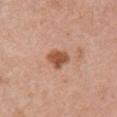workup — imaged on a skin check; not biopsied
patient — female, in their 50s
body site — the chest
image source — total-body-photography crop, ~15 mm field of view
automated lesion analysis — a classifier nevus-likeness of about 90/100 and a detector confidence of about 100 out of 100 that the crop contains a lesion
lesion size — about 3 mm